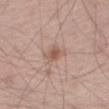Assessment: Captured during whole-body skin photography for melanoma surveillance; the lesion was not biopsied. Acquisition and patient details: A male patient approximately 60 years of age. An algorithmic analysis of the crop reported an eccentricity of roughly 0.7 and a symmetry-axis asymmetry near 0.3. It also reported a mean CIELAB color near L≈56 a*≈19 b*≈26, about 9 CIELAB-L* units darker than the surrounding skin, and a lesion-to-skin contrast of about 6.5 (normalized; higher = more distinct). The analysis additionally found a border-irregularity index near 3/10, a color-variation rating of about 1.5/10, and radial color variation of about 0.5. Imaged with white-light lighting. Cropped from a whole-body photographic skin survey; the tile spans about 15 mm. Longest diameter approximately 3 mm. The lesion is located on the right thigh.